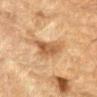Case summary:
* workup · catalogued during a skin exam; not biopsied
* acquisition · 15 mm crop, total-body photography
* automated lesion analysis · a lesion area of about 10 mm², an eccentricity of roughly 0.9, and a symmetry-axis asymmetry near 0.45; a lesion color around L≈50 a*≈18 b*≈34 in CIELAB and a lesion-to-skin contrast of about 7.5 (normalized; higher = more distinct); border irregularity of about 5 on a 0–10 scale, internal color variation of about 3 on a 0–10 scale, and a peripheral color-asymmetry measure near 1; a classifier nevus-likeness of about 0/100 and lesion-presence confidence of about 90/100
* site · the left forearm
* size · about 6 mm
* subject · male, approximately 85 years of age or older